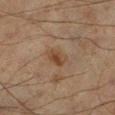No biopsy was performed on this lesion — it was imaged during a full skin examination and was not determined to be concerning.
The lesion is located on the right lower leg.
The recorded lesion diameter is about 2.5 mm.
A male subject in their mid-60s.
This image is a 15 mm lesion crop taken from a total-body photograph.
The total-body-photography lesion software estimated an area of roughly 4.5 mm², an outline eccentricity of about 0.65 (0 = round, 1 = elongated), and a shape-asymmetry score of about 0.2 (0 = symmetric). The analysis additionally found roughly 7 lightness units darker than nearby skin and a normalized border contrast of about 7.
This is a cross-polarized tile.